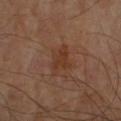The tile uses cross-polarized illumination. An algorithmic analysis of the crop reported a footprint of about 6.5 mm² and two-axis asymmetry of about 0.3. The software also gave roughly 6 lightness units darker than nearby skin and a normalized border contrast of about 6.5. The analysis additionally found a border-irregularity rating of about 4/10 and a within-lesion color-variation index near 2/10. It also reported an automated nevus-likeness rating near 0 out of 100 and a detector confidence of about 100 out of 100 that the crop contains a lesion. Approximately 4 mm at its widest. From the arm. This image is a 15 mm lesion crop taken from a total-body photograph. A male patient aged 68 to 72.